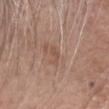No biopsy was performed on this lesion — it was imaged during a full skin examination and was not determined to be concerning. A male subject, aged 73 to 77. Located on the head or neck. Cropped from a whole-body photographic skin survey; the tile spans about 15 mm.